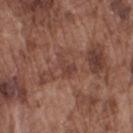Q: Was this lesion biopsied?
A: catalogued during a skin exam; not biopsied